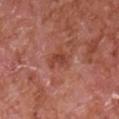Impression:
Imaged during a routine full-body skin examination; the lesion was not biopsied and no histopathology is available.
Context:
Captured under white-light illumination. A close-up tile cropped from a whole-body skin photograph, about 15 mm across. On the chest. A male patient aged 63 to 67. About 3 mm across.